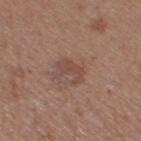Recorded during total-body skin imaging; not selected for excision or biopsy. A roughly 15 mm field-of-view crop from a total-body skin photograph. A female subject about 40 years old. From the right thigh.